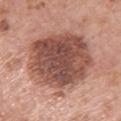biopsy_status: not biopsied; imaged during a skin examination
site: upper back
image:
  source: total-body photography crop
  field_of_view_mm: 15
patient:
  sex: female
  age_approx: 60
lesion_size:
  long_diameter_mm_approx: 8.5
lighting: white-light